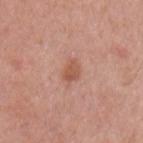Impression: The lesion was tiled from a total-body skin photograph and was not biopsied. Image and clinical context: Automated image analysis of the tile measured an area of roughly 4 mm². It also reported an average lesion color of about L≈55 a*≈24 b*≈30 (CIELAB) and a normalized border contrast of about 7. And it measured border irregularity of about 3 on a 0–10 scale, internal color variation of about 2.5 on a 0–10 scale, and a peripheral color-asymmetry measure near 0.5. It also reported a detector confidence of about 100 out of 100 that the crop contains a lesion. On the left upper arm. A 15 mm crop from a total-body photograph taken for skin-cancer surveillance. A male patient in their mid-50s. The recorded lesion diameter is about 2.5 mm.